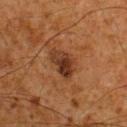The lesion was tiled from a total-body skin photograph and was not biopsied.
A lesion tile, about 15 mm wide, cut from a 3D total-body photograph.
The patient is a male about 60 years old.
The lesion is on the upper back.
Captured under cross-polarized illumination.
The lesion's longest dimension is about 4 mm.